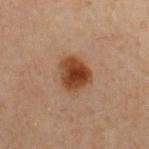Recorded during total-body skin imaging; not selected for excision or biopsy. The tile uses cross-polarized illumination. On the chest. Cropped from a whole-body photographic skin survey; the tile spans about 15 mm. A male patient, about 60 years old. Longest diameter approximately 4 mm.